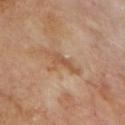Notes:
- follow-up: imaged on a skin check; not biopsied
- patient: male, aged 68–72
- acquisition: ~15 mm crop, total-body skin-cancer survey
- location: the chest